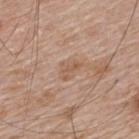This lesion was catalogued during total-body skin photography and was not selected for biopsy. This image is a 15 mm lesion crop taken from a total-body photograph. A male subject, roughly 65 years of age. The lesion is located on the upper back.